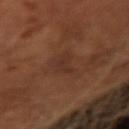Notes:
– notes — total-body-photography surveillance lesion; no biopsy
– automated lesion analysis — a footprint of about 3.5 mm², an eccentricity of roughly 0.85, and a shape-asymmetry score of about 0.55 (0 = symmetric); a nevus-likeness score of about 0/100 and lesion-presence confidence of about 95/100
– illumination — cross-polarized illumination
– patient — male, aged around 65
– lesion diameter — ≈3 mm
– image source — 15 mm crop, total-body photography